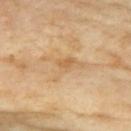Clinical impression: Recorded during total-body skin imaging; not selected for excision or biopsy. Context: An algorithmic analysis of the crop reported an outline eccentricity of about 0.85 (0 = round, 1 = elongated). The software also gave an average lesion color of about L≈64 a*≈17 b*≈39 (CIELAB) and a lesion-to-skin contrast of about 5.5 (normalized; higher = more distinct). A roughly 15 mm field-of-view crop from a total-body skin photograph. From the chest. Captured under cross-polarized illumination. A female patient aged 73 to 77. The recorded lesion diameter is about 5.5 mm.